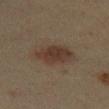Part of a total-body skin-imaging series; this lesion was reviewed on a skin check and was not flagged for biopsy. Longest diameter approximately 5 mm. A female subject, approximately 40 years of age. A close-up tile cropped from a whole-body skin photograph, about 15 mm across. This is a cross-polarized tile. From the left lower leg.